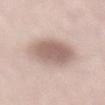Q: Was this lesion biopsied?
A: imaged on a skin check; not biopsied
Q: How was the tile lit?
A: white-light illumination
Q: How was this image acquired?
A: ~15 mm tile from a whole-body skin photo
Q: What is the anatomic site?
A: the back
Q: Patient demographics?
A: male, aged approximately 65